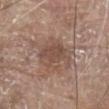follow-up — imaged on a skin check; not biopsied | body site — the front of the torso | automated lesion analysis — a footprint of about 14 mm², an outline eccentricity of about 0.65 (0 = round, 1 = elongated), and a shape-asymmetry score of about 0.3 (0 = symmetric); a mean CIELAB color near L≈49 a*≈17 b*≈25 and a lesion–skin lightness drop of about 8; a border-irregularity rating of about 5/10 and a peripheral color-asymmetry measure near 1; lesion-presence confidence of about 100/100 | lesion diameter — ≈5 mm | patient — male, in their 80s | image source — total-body-photography crop, ~15 mm field of view | lighting — white-light illumination.